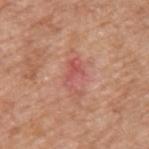Assessment:
Captured during whole-body skin photography for melanoma surveillance; the lesion was not biopsied.
Image and clinical context:
A close-up tile cropped from a whole-body skin photograph, about 15 mm across. Longest diameter approximately 4 mm. From the upper back. Automated image analysis of the tile measured a within-lesion color-variation index near 2.5/10 and peripheral color asymmetry of about 1. Captured under white-light illumination. A male subject, aged 58–62.